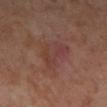Q: Was a biopsy performed?
A: no biopsy performed (imaged during a skin exam)
Q: Illumination type?
A: cross-polarized
Q: How large is the lesion?
A: ≈5 mm
Q: What are the patient's age and sex?
A: female, aged approximately 55
Q: Lesion location?
A: the right lower leg
Q: What kind of image is this?
A: 15 mm crop, total-body photography